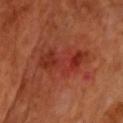Clinical impression: The lesion was photographed on a routine skin check and not biopsied; there is no pathology result. Clinical summary: Cropped from a total-body skin-imaging series; the visible field is about 15 mm. This is a cross-polarized tile. A male patient aged approximately 65. On the chest.